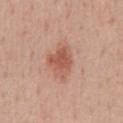The lesion was tiled from a total-body skin photograph and was not biopsied.
Imaged with white-light lighting.
A 15 mm crop from a total-body photograph taken for skin-cancer surveillance.
The lesion is on the chest.
A male patient aged approximately 50.